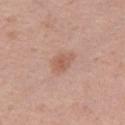Impression:
Recorded during total-body skin imaging; not selected for excision or biopsy.
Background:
Cropped from a total-body skin-imaging series; the visible field is about 15 mm. This is a white-light tile. The patient is a female aged 38 to 42. The lesion's longest dimension is about 3 mm. Located on the right thigh. Automated tile analysis of the lesion measured an area of roughly 5 mm², an outline eccentricity of about 0.7 (0 = round, 1 = elongated), and two-axis asymmetry of about 0.25. The analysis additionally found border irregularity of about 2.5 on a 0–10 scale, a color-variation rating of about 3/10, and radial color variation of about 1.